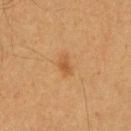{"biopsy_status": "not biopsied; imaged during a skin examination", "lighting": "cross-polarized", "patient": {"sex": "male", "age_approx": 60}, "automated_metrics": {"cielab_L": 51, "cielab_a": 21, "cielab_b": 38, "vs_skin_darker_L": 7.0, "vs_skin_contrast_norm": 5.5, "border_irregularity_0_10": 3.5, "color_variation_0_10": 2.5, "peripheral_color_asymmetry": 0.5}, "site": "chest", "image": {"source": "total-body photography crop", "field_of_view_mm": 15}}